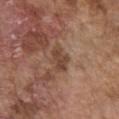Clinical impression: Imaged during a routine full-body skin examination; the lesion was not biopsied and no histopathology is available. Context: A male subject, approximately 75 years of age. The lesion-visualizer software estimated a mean CIELAB color near L≈44 a*≈19 b*≈28. It also reported an automated nevus-likeness rating near 0 out of 100 and a detector confidence of about 100 out of 100 that the crop contains a lesion. A 15 mm close-up tile from a total-body photography series done for melanoma screening. Measured at roughly 3.5 mm in maximum diameter. This is a white-light tile. The lesion is on the chest.